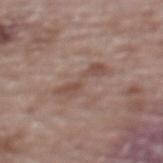Imaged during a routine full-body skin examination; the lesion was not biopsied and no histopathology is available. Imaged with white-light lighting. A female patient, approximately 65 years of age. The lesion is located on the upper back. Automated image analysis of the tile measured a shape eccentricity near 0.95 and a symmetry-axis asymmetry near 0.55. A region of skin cropped from a whole-body photographic capture, roughly 15 mm wide. Measured at roughly 5.5 mm in maximum diameter.